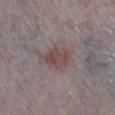This lesion was catalogued during total-body skin photography and was not selected for biopsy.